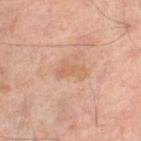Impression:
Part of a total-body skin-imaging series; this lesion was reviewed on a skin check and was not flagged for biopsy.
Acquisition and patient details:
The lesion is on the leg. This image is a 15 mm lesion crop taken from a total-body photograph. About 3.5 mm across. An algorithmic analysis of the crop reported an outline eccentricity of about 0.85 (0 = round, 1 = elongated) and two-axis asymmetry of about 0.35. The analysis additionally found an automated nevus-likeness rating near 0 out of 100 and a lesion-detection confidence of about 100/100. The tile uses cross-polarized illumination. A male subject, approximately 65 years of age.